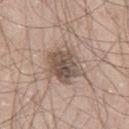Assessment:
Recorded during total-body skin imaging; not selected for excision or biopsy.
Context:
This image is a 15 mm lesion crop taken from a total-body photograph. The subject is a male approximately 60 years of age. The lesion is on the right thigh. Captured under white-light illumination. Approximately 4.5 mm at its widest.